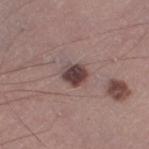Captured during whole-body skin photography for melanoma surveillance; the lesion was not biopsied.
Approximately 2.5 mm at its widest.
A male subject aged 43 to 47.
Located on the right thigh.
A 15 mm close-up extracted from a 3D total-body photography capture.
The tile uses white-light illumination.
The lesion-visualizer software estimated a mean CIELAB color near L≈40 a*≈17 b*≈18 and a normalized lesion–skin contrast near 11.5.